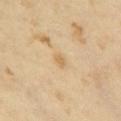The lesion was photographed on a routine skin check and not biopsied; there is no pathology result.
Cropped from a total-body skin-imaging series; the visible field is about 15 mm.
Approximately 2.5 mm at its widest.
The lesion-visualizer software estimated an eccentricity of roughly 0.75 and a shape-asymmetry score of about 0.15 (0 = symmetric). The software also gave a border-irregularity rating of about 1.5/10, internal color variation of about 2.5 on a 0–10 scale, and peripheral color asymmetry of about 1.
A female subject aged around 35.
From the chest.
Imaged with cross-polarized lighting.